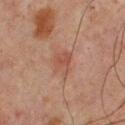patient: male, roughly 65 years of age | TBP lesion metrics: an automated nevus-likeness rating near 5 out of 100 and a detector confidence of about 100 out of 100 that the crop contains a lesion | anatomic site: the upper back | imaging modality: total-body-photography crop, ~15 mm field of view | tile lighting: cross-polarized | diameter: about 3 mm.